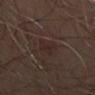notes — no biopsy performed (imaged during a skin exam)
TBP lesion metrics — a lesion area of about 4.5 mm², an outline eccentricity of about 0.85 (0 = round, 1 = elongated), and two-axis asymmetry of about 0.4; a border-irregularity index near 5/10, internal color variation of about 1 on a 0–10 scale, and peripheral color asymmetry of about 0.5; a nevus-likeness score of about 0/100 and a detector confidence of about 85 out of 100 that the crop contains a lesion
anatomic site — the mid back
patient — male, in their 50s
acquisition — total-body-photography crop, ~15 mm field of view